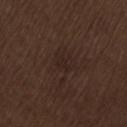The lesion was tiled from a total-body skin photograph and was not biopsied. About 2.5 mm across. Located on the lower back. A male patient aged 68–72. An algorithmic analysis of the crop reported a footprint of about 2.5 mm², an outline eccentricity of about 0.9 (0 = round, 1 = elongated), and two-axis asymmetry of about 0.45. It also reported a border-irregularity rating of about 4.5/10, a color-variation rating of about 0/10, and peripheral color asymmetry of about 0. And it measured a nevus-likeness score of about 0/100 and a lesion-detection confidence of about 85/100. Captured under white-light illumination. This image is a 15 mm lesion crop taken from a total-body photograph.